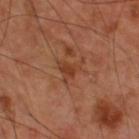* notes · catalogued during a skin exam; not biopsied